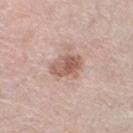{"biopsy_status": "not biopsied; imaged during a skin examination"}